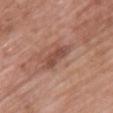image: 15 mm crop, total-body photography | automated lesion analysis: a lesion area of about 5.5 mm²; internal color variation of about 1.5 on a 0–10 scale; an automated nevus-likeness rating near 0 out of 100 | anatomic site: the upper back | size: ~4.5 mm (longest diameter) | illumination: white-light | patient: female, approximately 65 years of age.